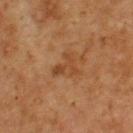  biopsy_status: not biopsied; imaged during a skin examination
  image:
    source: total-body photography crop
    field_of_view_mm: 15
  patient:
    sex: male
    age_approx: 60
  lesion_size:
    long_diameter_mm_approx: 3.5
  site: upper back